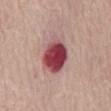Clinical impression: Recorded during total-body skin imaging; not selected for excision or biopsy. Clinical summary: Cropped from a total-body skin-imaging series; the visible field is about 15 mm. Longest diameter approximately 4.5 mm. A female subject, in their mid- to late 60s. Captured under white-light illumination. On the mid back.